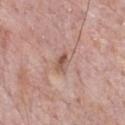  biopsy_status: not biopsied; imaged during a skin examination
  patient:
    sex: male
    age_approx: 70
  image:
    source: total-body photography crop
    field_of_view_mm: 15
  lighting: white-light
  automated_metrics:
    border_irregularity_0_10: 2.0
    color_variation_0_10: 4.5
    peripheral_color_asymmetry: 1.5
    nevus_likeness_0_100: 5
    lesion_detection_confidence_0_100: 100
  site: front of the torso